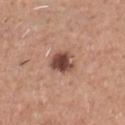Notes:
– workup · imaged on a skin check; not biopsied